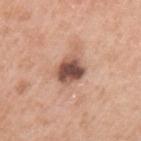Imaged during a routine full-body skin examination; the lesion was not biopsied and no histopathology is available.
A region of skin cropped from a whole-body photographic capture, roughly 15 mm wide.
The lesion's longest dimension is about 3 mm.
A male subject, roughly 60 years of age.
The tile uses white-light illumination.
On the arm.
Automated image analysis of the tile measured a footprint of about 8 mm² and a symmetry-axis asymmetry near 0.25. The analysis additionally found a mean CIELAB color near L≈51 a*≈21 b*≈26 and a normalized lesion–skin contrast near 11.5. And it measured a border-irregularity rating of about 2/10 and a peripheral color-asymmetry measure near 2.